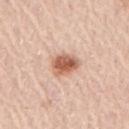anatomic site — the right upper arm
patient — male, aged 63 to 67
acquisition — ~15 mm crop, total-body skin-cancer survey
lesion diameter — ≈3.5 mm
automated lesion analysis — a mean CIELAB color near L≈61 a*≈23 b*≈32, about 15 CIELAB-L* units darker than the surrounding skin, and a lesion-to-skin contrast of about 9.5 (normalized; higher = more distinct)
lighting — white-light illumination